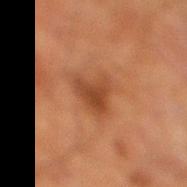{
  "biopsy_status": "not biopsied; imaged during a skin examination",
  "automated_metrics": {
    "cielab_L": 37,
    "cielab_a": 22,
    "cielab_b": 31,
    "vs_skin_darker_L": 8.0,
    "nevus_likeness_0_100": 35
  },
  "site": "leg",
  "patient": {
    "sex": "male",
    "age_approx": 70
  },
  "image": {
    "source": "total-body photography crop",
    "field_of_view_mm": 15
  },
  "lighting": "cross-polarized"
}